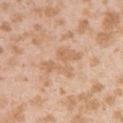Recorded during total-body skin imaging; not selected for excision or biopsy. The subject is a female in their mid- to late 20s. On the arm. This is a white-light tile. A region of skin cropped from a whole-body photographic capture, roughly 15 mm wide. The lesion's longest dimension is about 5 mm.